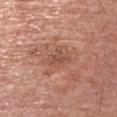Q: Was a biopsy performed?
A: catalogued during a skin exam; not biopsied
Q: Illumination type?
A: white-light illumination
Q: What is the lesion's diameter?
A: ≈4 mm
Q: What is the anatomic site?
A: the left upper arm
Q: Patient demographics?
A: male, approximately 75 years of age
Q: What did automated image analysis measure?
A: an average lesion color of about L≈52 a*≈23 b*≈29 (CIELAB) and a normalized border contrast of about 5.5
Q: What is the imaging modality?
A: ~15 mm tile from a whole-body skin photo